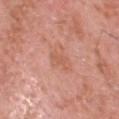follow-up = imaged on a skin check; not biopsied | site = the head or neck | subject = male, roughly 55 years of age | acquisition = 15 mm crop, total-body photography | lighting = white-light.